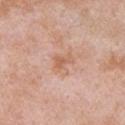| key | value |
|---|---|
| biopsy status | catalogued during a skin exam; not biopsied |
| image source | 15 mm crop, total-body photography |
| illumination | white-light illumination |
| subject | male, aged 53–57 |
| automated metrics | a lesion area of about 5.5 mm², an eccentricity of roughly 0.6, and two-axis asymmetry of about 0.45; a mean CIELAB color near L≈62 a*≈22 b*≈31, roughly 8 lightness units darker than nearby skin, and a lesion-to-skin contrast of about 6 (normalized; higher = more distinct); a classifier nevus-likeness of about 0/100 and lesion-presence confidence of about 100/100 |
| anatomic site | the left upper arm |
| lesion size | about 3 mm |